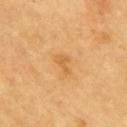Context: The lesion is on the upper back. Imaged with cross-polarized lighting. Cropped from a total-body skin-imaging series; the visible field is about 15 mm. An algorithmic analysis of the crop reported a mean CIELAB color near L≈62 a*≈23 b*≈47. It also reported an automated nevus-likeness rating near 5 out of 100 and lesion-presence confidence of about 100/100. The recorded lesion diameter is about 2.5 mm. A male patient approximately 65 years of age.